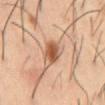Imaged during a routine full-body skin examination; the lesion was not biopsied and no histopathology is available.
An algorithmic analysis of the crop reported an area of roughly 6.5 mm². The analysis additionally found a lesion color around L≈54 a*≈22 b*≈33 in CIELAB and about 14 CIELAB-L* units darker than the surrounding skin. And it measured a border-irregularity rating of about 2/10, internal color variation of about 5 on a 0–10 scale, and peripheral color asymmetry of about 1.5.
The recorded lesion diameter is about 3.5 mm.
A 15 mm crop from a total-body photograph taken for skin-cancer surveillance.
From the abdomen.
A male subject, in their mid- to late 50s.
The tile uses cross-polarized illumination.